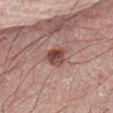<tbp_lesion>
  <biopsy_status>not biopsied; imaged during a skin examination</biopsy_status>
  <site>abdomen</site>
  <automated_metrics>
    <cielab_L>46</cielab_L>
    <cielab_a>22</cielab_a>
    <cielab_b>23</cielab_b>
    <vs_skin_darker_L>13.0</vs_skin_darker_L>
    <vs_skin_contrast_norm>9.5</vs_skin_contrast_norm>
    <nevus_likeness_0_100>90</nevus_likeness_0_100>
    <lesion_detection_confidence_0_100>100</lesion_detection_confidence_0_100>
  </automated_metrics>
  <patient>
    <sex>female</sex>
    <age_approx>65</age_approx>
  </patient>
  <lesion_size>
    <long_diameter_mm_approx>3.0</long_diameter_mm_approx>
  </lesion_size>
  <image>
    <source>total-body photography crop</source>
    <field_of_view_mm>15</field_of_view_mm>
  </image>
</tbp_lesion>